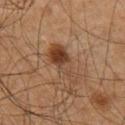| key | value |
|---|---|
| diameter | about 5.5 mm |
| acquisition | 15 mm crop, total-body photography |
| tile lighting | cross-polarized illumination |
| TBP lesion metrics | a footprint of about 9.5 mm², a shape eccentricity near 0.9, and two-axis asymmetry of about 0.45 |
| patient | male, aged 58 to 62 |
| body site | the right thigh |